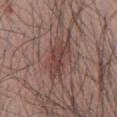No biopsy was performed on this lesion — it was imaged during a full skin examination and was not determined to be concerning. Located on the front of the torso. The total-body-photography lesion software estimated a lesion color around L≈43 a*≈20 b*≈22 in CIELAB, about 8 CIELAB-L* units darker than the surrounding skin, and a lesion-to-skin contrast of about 7 (normalized; higher = more distinct). A roughly 15 mm field-of-view crop from a total-body skin photograph. A male patient aged 38–42.